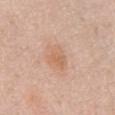biopsy_status: not biopsied; imaged during a skin examination
site: abdomen
automated_metrics:
  eccentricity: 0.65
  shape_asymmetry: 0.2
  cielab_L: 64
  cielab_a: 20
  cielab_b: 32
  vs_skin_darker_L: 7.0
  vs_skin_contrast_norm: 5.5
  nevus_likeness_0_100: 10
  lesion_detection_confidence_0_100: 100
patient:
  sex: male
  age_approx: 80
image:
  source: total-body photography crop
  field_of_view_mm: 15
lighting: white-light
lesion_size:
  long_diameter_mm_approx: 3.0